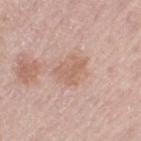This lesion was catalogued during total-body skin photography and was not selected for biopsy. A 15 mm close-up extracted from a 3D total-body photography capture. The patient is a female aged approximately 65. Located on the left thigh.